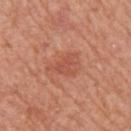Case summary:
• follow-up · no biopsy performed (imaged during a skin exam)
• diameter · ~4 mm (longest diameter)
• image · ~15 mm tile from a whole-body skin photo
• body site · the right upper arm
• lighting · white-light illumination
• subject · female, aged approximately 75
• TBP lesion metrics · a mean CIELAB color near L≈53 a*≈28 b*≈33, about 7 CIELAB-L* units darker than the surrounding skin, and a normalized lesion–skin contrast near 5; border irregularity of about 4.5 on a 0–10 scale, internal color variation of about 2 on a 0–10 scale, and peripheral color asymmetry of about 0.5